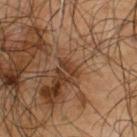Clinical impression:
The lesion was photographed on a routine skin check and not biopsied; there is no pathology result.
Background:
The lesion's longest dimension is about 2.5 mm. On the upper back. The tile uses cross-polarized illumination. A male patient about 65 years old. A close-up tile cropped from a whole-body skin photograph, about 15 mm across.